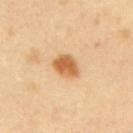<tbp_lesion>
<biopsy_status>not biopsied; imaged during a skin examination</biopsy_status>
<lesion_size>
  <long_diameter_mm_approx>3.5</long_diameter_mm_approx>
</lesion_size>
<site>mid back</site>
<lighting>cross-polarized</lighting>
<patient>
  <sex>male</sex>
  <age_approx>40</age_approx>
</patient>
<image>
  <source>total-body photography crop</source>
  <field_of_view_mm>15</field_of_view_mm>
</image>
</tbp_lesion>